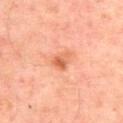Impression:
No biopsy was performed on this lesion — it was imaged during a full skin examination and was not determined to be concerning.
Acquisition and patient details:
The subject is a male approximately 50 years of age. Captured under cross-polarized illumination. The lesion's longest dimension is about 3 mm. This image is a 15 mm lesion crop taken from a total-body photograph. An algorithmic analysis of the crop reported an average lesion color of about L≈64 a*≈31 b*≈39 (CIELAB), about 11 CIELAB-L* units darker than the surrounding skin, and a normalized lesion–skin contrast near 7. It also reported a border-irregularity index near 3/10, internal color variation of about 6.5 on a 0–10 scale, and peripheral color asymmetry of about 2. And it measured an automated nevus-likeness rating near 30 out of 100. On the back.